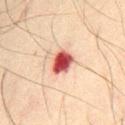Impression:
Captured during whole-body skin photography for melanoma surveillance; the lesion was not biopsied.
Acquisition and patient details:
The lesion-visualizer software estimated a mean CIELAB color near L≈46 a*≈29 b*≈25, a lesion–skin lightness drop of about 19, and a lesion-to-skin contrast of about 13 (normalized; higher = more distinct). The analysis additionally found a lesion-detection confidence of about 100/100. A male subject, in their mid-40s. Located on the abdomen. The lesion's longest dimension is about 3.5 mm. A roughly 15 mm field-of-view crop from a total-body skin photograph.